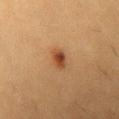- workup: total-body-photography surveillance lesion; no biopsy
- lesion diameter: about 2.5 mm
- imaging modality: 15 mm crop, total-body photography
- body site: the chest
- automated lesion analysis: border irregularity of about 2.5 on a 0–10 scale and radial color variation of about 1.5; an automated nevus-likeness rating near 100 out of 100 and a detector confidence of about 100 out of 100 that the crop contains a lesion
- patient: female, aged 18–22
- tile lighting: cross-polarized